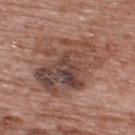Notes:
* follow-up: imaged on a skin check; not biopsied
* image source: total-body-photography crop, ~15 mm field of view
* anatomic site: the upper back
* subject: male, about 70 years old